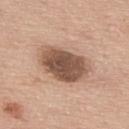Findings:
- follow-up: catalogued during a skin exam; not biopsied
- patient: male, aged approximately 75
- lighting: white-light illumination
- acquisition: 15 mm crop, total-body photography
- anatomic site: the upper back
- size: ≈6 mm